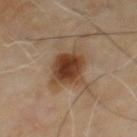No biopsy was performed on this lesion — it was imaged during a full skin examination and was not determined to be concerning. The lesion-visualizer software estimated a symmetry-axis asymmetry near 0.2. And it measured a lesion color around L≈39 a*≈18 b*≈30 in CIELAB and a lesion–skin lightness drop of about 13. The analysis additionally found a border-irregularity rating of about 2.5/10, a within-lesion color-variation index near 6/10, and radial color variation of about 1.5. It also reported an automated nevus-likeness rating near 95 out of 100 and a detector confidence of about 100 out of 100 that the crop contains a lesion. A male subject aged around 60. Approximately 5 mm at its widest. The lesion is on the mid back. Imaged with cross-polarized lighting. A 15 mm close-up tile from a total-body photography series done for melanoma screening.